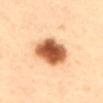Clinical impression: Recorded during total-body skin imaging; not selected for excision or biopsy. Acquisition and patient details: Approximately 5 mm at its widest. The lesion-visualizer software estimated a footprint of about 16 mm², an eccentricity of roughly 0.65, and two-axis asymmetry of about 0.15. The tile uses cross-polarized illumination. A female patient, aged 38–42. Cropped from a total-body skin-imaging series; the visible field is about 15 mm. Located on the upper back.